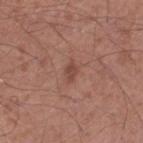notes: total-body-photography surveillance lesion; no biopsy | patient: male, aged approximately 55 | acquisition: 15 mm crop, total-body photography | site: the left thigh.